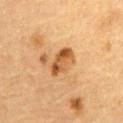Clinical impression:
The lesion was photographed on a routine skin check and not biopsied; there is no pathology result.
Background:
Automated image analysis of the tile measured a footprint of about 7 mm² and an eccentricity of roughly 0.85. It also reported roughly 12 lightness units darker than nearby skin and a normalized border contrast of about 8.5. It also reported a nevus-likeness score of about 50/100. Located on the abdomen. A lesion tile, about 15 mm wide, cut from a 3D total-body photograph. Captured under cross-polarized illumination. A male subject, in their mid- to late 80s or older. Longest diameter approximately 4 mm.